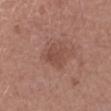biopsy_status: not biopsied; imaged during a skin examination
image:
  source: total-body photography crop
  field_of_view_mm: 15
patient:
  sex: female
  age_approx: 45
lighting: white-light
site: arm